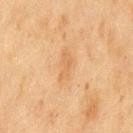Imaged during a routine full-body skin examination; the lesion was not biopsied and no histopathology is available. A male patient aged approximately 75. Cropped from a total-body skin-imaging series; the visible field is about 15 mm. On the chest.